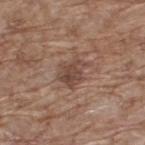Q: Was this lesion biopsied?
A: no biopsy performed (imaged during a skin exam)
Q: Automated lesion metrics?
A: a shape eccentricity near 0.6 and a symmetry-axis asymmetry near 0.3
Q: Patient demographics?
A: male, approximately 70 years of age
Q: What kind of image is this?
A: total-body-photography crop, ~15 mm field of view
Q: What is the anatomic site?
A: the upper back
Q: What lighting was used for the tile?
A: white-light
Q: Lesion size?
A: ≈3.5 mm